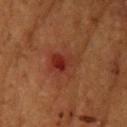Q: Was this lesion biopsied?
A: total-body-photography surveillance lesion; no biopsy
Q: What lighting was used for the tile?
A: cross-polarized illumination
Q: What is the anatomic site?
A: the left upper arm
Q: What are the patient's age and sex?
A: female, about 80 years old
Q: How was this image acquired?
A: 15 mm crop, total-body photography